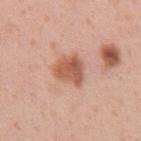Part of a total-body skin-imaging series; this lesion was reviewed on a skin check and was not flagged for biopsy.
A male patient, aged 53–57.
Approximately 4 mm at its widest.
On the abdomen.
This is a white-light tile.
A roughly 15 mm field-of-view crop from a total-body skin photograph.